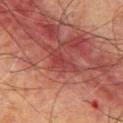Part of a total-body skin-imaging series; this lesion was reviewed on a skin check and was not flagged for biopsy. A male patient, aged around 65. Automated tile analysis of the lesion measured a lesion area of about 5.5 mm², a shape eccentricity near 0.85, and two-axis asymmetry of about 0.45. The analysis additionally found a mean CIELAB color near L≈33 a*≈27 b*≈22, roughly 6 lightness units darker than nearby skin, and a normalized border contrast of about 5.5. The software also gave border irregularity of about 5.5 on a 0–10 scale, a color-variation rating of about 2/10, and a peripheral color-asymmetry measure near 0.5. And it measured a classifier nevus-likeness of about 0/100 and a lesion-detection confidence of about 75/100. Measured at roughly 4 mm in maximum diameter. From the chest. Cropped from a total-body skin-imaging series; the visible field is about 15 mm.